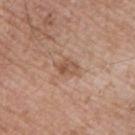{"biopsy_status": "not biopsied; imaged during a skin examination", "lighting": "white-light", "automated_metrics": {"area_mm2_approx": 4.0, "eccentricity": 0.7, "cielab_L": 53, "cielab_a": 20, "cielab_b": 30, "vs_skin_darker_L": 8.0, "border_irregularity_0_10": 2.0, "peripheral_color_asymmetry": 2.0, "nevus_likeness_0_100": 0, "lesion_detection_confidence_0_100": 100}, "patient": {"sex": "male", "age_approx": 65}, "image": {"source": "total-body photography crop", "field_of_view_mm": 15}, "site": "chest", "lesion_size": {"long_diameter_mm_approx": 2.5}}